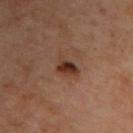{"biopsy_status": "not biopsied; imaged during a skin examination", "lesion_size": {"long_diameter_mm_approx": 3.5}, "image": {"source": "total-body photography crop", "field_of_view_mm": 15}, "lighting": "cross-polarized", "site": "right upper arm", "patient": {"sex": "male", "age_approx": 70}}